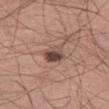  biopsy_status: not biopsied; imaged during a skin examination